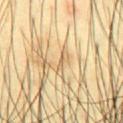Imaged during a routine full-body skin examination; the lesion was not biopsied and no histopathology is available.
Approximately 1.5 mm at its widest.
The lesion is located on the abdomen.
This is a cross-polarized tile.
A region of skin cropped from a whole-body photographic capture, roughly 15 mm wide.
A male patient, roughly 45 years of age.